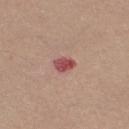Q: Is there a histopathology result?
A: imaged on a skin check; not biopsied
Q: What did automated image analysis measure?
A: a mean CIELAB color near L≈51 a*≈29 b*≈23, roughly 13 lightness units darker than nearby skin, and a normalized lesion–skin contrast near 9
Q: Lesion location?
A: the right thigh
Q: What lighting was used for the tile?
A: white-light illumination
Q: What are the patient's age and sex?
A: female, aged approximately 45
Q: What kind of image is this?
A: ~15 mm tile from a whole-body skin photo